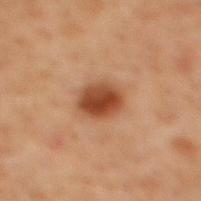notes=catalogued during a skin exam; not biopsied | patient=male, approximately 70 years of age | anatomic site=the mid back | acquisition=~15 mm tile from a whole-body skin photo.